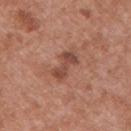workup = total-body-photography surveillance lesion; no biopsy